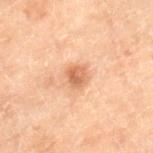The subject is a male aged 68 to 72.
This image is a 15 mm lesion crop taken from a total-body photograph.
On the right lower leg.
The lesion's longest dimension is about 2.5 mm.